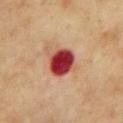biopsy status = imaged on a skin check; not biopsied
body site = the chest
patient = male, about 70 years old
imaging modality = 15 mm crop, total-body photography